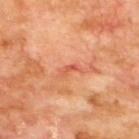This lesion was catalogued during total-body skin photography and was not selected for biopsy. A 15 mm close-up extracted from a 3D total-body photography capture. A male patient aged approximately 70. The lesion is located on the upper back. Automated image analysis of the tile measured a footprint of about 2 mm², an eccentricity of roughly 0.9, and a symmetry-axis asymmetry near 0.5. It also reported a mean CIELAB color near L≈57 a*≈34 b*≈37, a lesion–skin lightness drop of about 9, and a normalized border contrast of about 5.5. And it measured a border-irregularity index near 5/10 and a within-lesion color-variation index near 0/10.